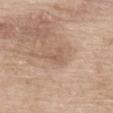follow-up=total-body-photography surveillance lesion; no biopsy
subject=female, in their mid- to late 70s
lesion diameter=~3 mm (longest diameter)
imaging modality=15 mm crop, total-body photography
illumination=white-light
location=the mid back